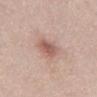The lesion was photographed on a routine skin check and not biopsied; there is no pathology result.
About 3 mm across.
Automated tile analysis of the lesion measured a lesion area of about 5.5 mm² and a symmetry-axis asymmetry near 0.2. The software also gave a mean CIELAB color near L≈57 a*≈21 b*≈25, roughly 11 lightness units darker than nearby skin, and a normalized lesion–skin contrast near 7.
Imaged with white-light lighting.
A female patient, about 30 years old.
From the abdomen.
A 15 mm close-up tile from a total-body photography series done for melanoma screening.